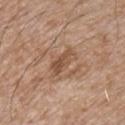Case summary:
– follow-up · catalogued during a skin exam; not biopsied
– patient · male, aged around 65
– tile lighting · white-light illumination
– lesion diameter · ~4 mm (longest diameter)
– TBP lesion metrics · a footprint of about 6.5 mm², a shape eccentricity near 0.85, and a shape-asymmetry score of about 0.25 (0 = symmetric); a border-irregularity index near 3/10 and peripheral color asymmetry of about 1.5
– site · the chest
– image source · ~15 mm tile from a whole-body skin photo